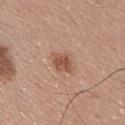workup: total-body-photography surveillance lesion; no biopsy
size: about 3 mm
body site: the chest
acquisition: total-body-photography crop, ~15 mm field of view
patient: male, about 25 years old